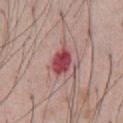Findings:
- workup — catalogued during a skin exam; not biopsied
- lighting — white-light illumination
- automated metrics — a lesion area of about 7 mm², a shape eccentricity near 0.7, and a symmetry-axis asymmetry near 0.15; an average lesion color of about L≈46 a*≈34 b*≈20 (CIELAB), a lesion–skin lightness drop of about 16, and a normalized border contrast of about 11; an automated nevus-likeness rating near 0 out of 100 and a lesion-detection confidence of about 100/100
- patient — male, roughly 55 years of age
- site — the front of the torso
- image source — total-body-photography crop, ~15 mm field of view